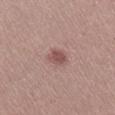biopsy_status: not biopsied; imaged during a skin examination
patient:
  sex: female
  age_approx: 20
image:
  source: total-body photography crop
  field_of_view_mm: 15
site: right lower leg